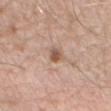workup: catalogued during a skin exam; not biopsied
anatomic site: the right forearm
acquisition: total-body-photography crop, ~15 mm field of view
patient: male, about 50 years old
illumination: white-light
TBP lesion metrics: a lesion color around L≈55 a*≈19 b*≈28 in CIELAB and a normalized lesion–skin contrast near 8; internal color variation of about 3 on a 0–10 scale and peripheral color asymmetry of about 1
lesion size: about 2.5 mm